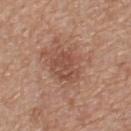Captured during whole-body skin photography for melanoma surveillance; the lesion was not biopsied.
This is a white-light tile.
Cropped from a whole-body photographic skin survey; the tile spans about 15 mm.
The recorded lesion diameter is about 7.5 mm.
A male subject, aged 53–57.
On the upper back.